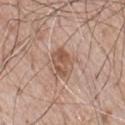Imaged during a routine full-body skin examination; the lesion was not biopsied and no histopathology is available.
Imaged with white-light lighting.
A male subject aged approximately 80.
About 4 mm across.
The lesion is on the chest.
A close-up tile cropped from a whole-body skin photograph, about 15 mm across.